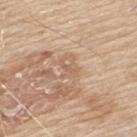notes: total-body-photography surveillance lesion; no biopsy | diameter: ≈2.5 mm | anatomic site: the back | patient: male, roughly 80 years of age | tile lighting: white-light | imaging modality: ~15 mm crop, total-body skin-cancer survey.